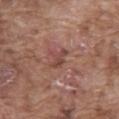Imaged during a routine full-body skin examination; the lesion was not biopsied and no histopathology is available.
The subject is a male aged around 75.
Measured at roughly 3 mm in maximum diameter.
The lesion-visualizer software estimated a lesion color around L≈45 a*≈23 b*≈24 in CIELAB, a lesion–skin lightness drop of about 8, and a normalized border contrast of about 6.5. And it measured a nevus-likeness score of about 10/100.
Imaged with white-light lighting.
Located on the abdomen.
A roughly 15 mm field-of-view crop from a total-body skin photograph.